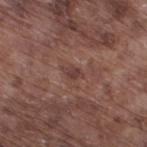Imaged during a routine full-body skin examination; the lesion was not biopsied and no histopathology is available.
Longest diameter approximately 2.5 mm.
The lesion is located on the right thigh.
A male subject, roughly 75 years of age.
This is a white-light tile.
Cropped from a whole-body photographic skin survey; the tile spans about 15 mm.